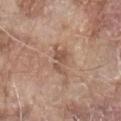On the front of the torso. A roughly 15 mm field-of-view crop from a total-body skin photograph. The subject is a male aged 78 to 82.